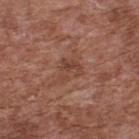Q: Was this lesion biopsied?
A: imaged on a skin check; not biopsied
Q: Patient demographics?
A: male, in their mid- to late 70s
Q: What kind of image is this?
A: 15 mm crop, total-body photography
Q: What is the anatomic site?
A: the back